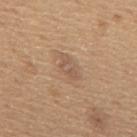notes: no biopsy performed (imaged during a skin exam) | patient: male, in their mid-60s | automated metrics: an area of roughly 6 mm² and a shape-asymmetry score of about 0.3 (0 = symmetric); a lesion–skin lightness drop of about 7; a border-irregularity rating of about 3.5/10, a color-variation rating of about 3/10, and peripheral color asymmetry of about 1 | diameter: about 4 mm | body site: the mid back | acquisition: 15 mm crop, total-body photography | illumination: white-light.